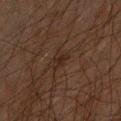{"lighting": "cross-polarized", "site": "right forearm", "automated_metrics": {"area_mm2_approx": 3.0, "eccentricity": 0.85, "shape_asymmetry": 0.3, "border_irregularity_0_10": 3.0, "color_variation_0_10": 0.5, "peripheral_color_asymmetry": 0.0}, "lesion_size": {"long_diameter_mm_approx": 2.5}, "patient": {"sex": "male", "age_approx": 60}, "image": {"source": "total-body photography crop", "field_of_view_mm": 15}}